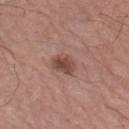  biopsy_status: not biopsied; imaged during a skin examination
  lighting: white-light
  lesion_size:
    long_diameter_mm_approx: 3.0
  automated_metrics:
    area_mm2_approx: 5.0
    eccentricity: 0.65
    shape_asymmetry: 0.25
  patient:
    sex: male
    age_approx: 65
  image:
    source: total-body photography crop
    field_of_view_mm: 15
  site: left forearm